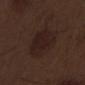Impression: This lesion was catalogued during total-body skin photography and was not selected for biopsy. Clinical summary: The total-body-photography lesion software estimated a lesion area of about 16 mm² and an outline eccentricity of about 0.45 (0 = round, 1 = elongated). It also reported a mean CIELAB color near L≈19 a*≈14 b*≈17 and roughly 5 lightness units darker than nearby skin. It also reported border irregularity of about 2 on a 0–10 scale, a color-variation rating of about 2/10, and peripheral color asymmetry of about 0.5. It also reported an automated nevus-likeness rating near 25 out of 100. The lesion is on the right thigh. Imaged with white-light lighting. The recorded lesion diameter is about 4.5 mm. A 15 mm crop from a total-body photograph taken for skin-cancer surveillance. A male subject, in their 70s.